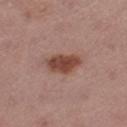Part of a total-body skin-imaging series; this lesion was reviewed on a skin check and was not flagged for biopsy. A close-up tile cropped from a whole-body skin photograph, about 15 mm across. The subject is a female in their mid-50s. The lesion's longest dimension is about 4 mm. The total-body-photography lesion software estimated a footprint of about 8 mm², an outline eccentricity of about 0.8 (0 = round, 1 = elongated), and a shape-asymmetry score of about 0.2 (0 = symmetric). Located on the leg. The tile uses white-light illumination.